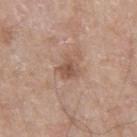{
  "biopsy_status": "not biopsied; imaged during a skin examination",
  "lighting": "white-light",
  "image": {
    "source": "total-body photography crop",
    "field_of_view_mm": 15
  },
  "automated_metrics": {
    "area_mm2_approx": 4.5,
    "shape_asymmetry": 0.25,
    "color_variation_0_10": 3.5,
    "peripheral_color_asymmetry": 1.5
  },
  "patient": {
    "sex": "male",
    "age_approx": 80
  },
  "site": "right thigh"
}